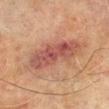Q: Was a biopsy performed?
A: imaged on a skin check; not biopsied
Q: Patient demographics?
A: male, roughly 70 years of age
Q: How large is the lesion?
A: ~9 mm (longest diameter)
Q: Automated lesion metrics?
A: a shape eccentricity near 0.95; a border-irregularity index near 3.5/10, a color-variation rating of about 5/10, and peripheral color asymmetry of about 1.5; a nevus-likeness score of about 20/100 and a lesion-detection confidence of about 100/100
Q: Where on the body is the lesion?
A: the left lower leg
Q: What is the imaging modality?
A: total-body-photography crop, ~15 mm field of view